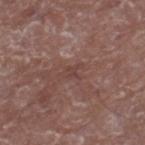Findings:
- image source: 15 mm crop, total-body photography
- lesion diameter: ≈2.5 mm
- illumination: white-light
- patient: male, about 65 years old
- anatomic site: the leg